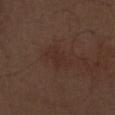No biopsy was performed on this lesion — it was imaged during a full skin examination and was not determined to be concerning. Captured under white-light illumination. A 15 mm close-up extracted from a 3D total-body photography capture. The recorded lesion diameter is about 3.5 mm. A male patient aged around 70. The lesion is on the right thigh.